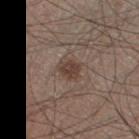Impression:
Imaged during a routine full-body skin examination; the lesion was not biopsied and no histopathology is available.
Background:
The subject is a male in their mid- to late 40s. Cropped from a total-body skin-imaging series; the visible field is about 15 mm. Captured under white-light illumination. Automated image analysis of the tile measured a lesion area of about 4.5 mm², a shape eccentricity near 0.45, and a symmetry-axis asymmetry near 0.25. It also reported a mean CIELAB color near L≈40 a*≈16 b*≈23, a lesion–skin lightness drop of about 9, and a lesion-to-skin contrast of about 7.5 (normalized; higher = more distinct). It also reported a border-irregularity index near 2.5/10 and internal color variation of about 2 on a 0–10 scale. Longest diameter approximately 2.5 mm. The lesion is located on the leg.